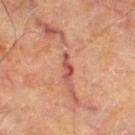Q: Is there a histopathology result?
A: imaged on a skin check; not biopsied
Q: How large is the lesion?
A: ~3 mm (longest diameter)
Q: How was this image acquired?
A: ~15 mm tile from a whole-body skin photo
Q: What is the anatomic site?
A: the right thigh
Q: Patient demographics?
A: aged 63 to 67